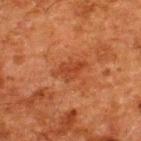Clinical impression: No biopsy was performed on this lesion — it was imaged during a full skin examination and was not determined to be concerning. Context: This is a cross-polarized tile. The lesion-visualizer software estimated an area of roughly 5.5 mm², a shape eccentricity near 0.85, and a shape-asymmetry score of about 0.3 (0 = symmetric). The software also gave roughly 6 lightness units darker than nearby skin and a normalized lesion–skin contrast near 6. It also reported border irregularity of about 3.5 on a 0–10 scale, a color-variation rating of about 1.5/10, and radial color variation of about 0.5. And it measured a nevus-likeness score of about 0/100 and a detector confidence of about 100 out of 100 that the crop contains a lesion. The lesion is located on the upper back. A region of skin cropped from a whole-body photographic capture, roughly 15 mm wide. A male patient, aged around 60. The lesion's longest dimension is about 4 mm.